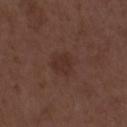No biopsy was performed on this lesion — it was imaged during a full skin examination and was not determined to be concerning.
An algorithmic analysis of the crop reported an average lesion color of about L≈31 a*≈18 b*≈22 (CIELAB) and roughly 5 lightness units darker than nearby skin.
A male patient aged around 50.
A region of skin cropped from a whole-body photographic capture, roughly 15 mm wide.
The recorded lesion diameter is about 4 mm.